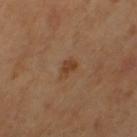notes = imaged on a skin check; not biopsied
size = ~2.5 mm (longest diameter)
subject = female, roughly 55 years of age
illumination = cross-polarized
imaging modality = ~15 mm crop, total-body skin-cancer survey
site = the left thigh